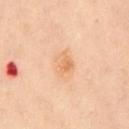Impression:
The lesion was tiled from a total-body skin photograph and was not biopsied.
Image and clinical context:
On the back. Cropped from a whole-body photographic skin survey; the tile spans about 15 mm. A male subject, in their 50s.